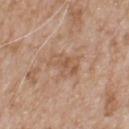{
  "biopsy_status": "not biopsied; imaged during a skin examination",
  "patient": {
    "sex": "male",
    "age_approx": 80
  },
  "lighting": "white-light",
  "automated_metrics": {
    "border_irregularity_0_10": 4.0,
    "color_variation_0_10": 3.5,
    "peripheral_color_asymmetry": 1.0,
    "nevus_likeness_0_100": 0,
    "lesion_detection_confidence_0_100": 100
  },
  "lesion_size": {
    "long_diameter_mm_approx": 4.0
  },
  "site": "upper back",
  "image": {
    "source": "total-body photography crop",
    "field_of_view_mm": 15
  }
}